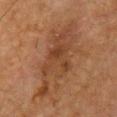The lesion was photographed on a routine skin check and not biopsied; there is no pathology result. This is a cross-polarized tile. Cropped from a total-body skin-imaging series; the visible field is about 15 mm. Automated image analysis of the tile measured a lesion area of about 33 mm², a shape eccentricity near 0.9, and a symmetry-axis asymmetry near 0.25. It also reported a mean CIELAB color near L≈34 a*≈17 b*≈27, a lesion–skin lightness drop of about 6, and a lesion-to-skin contrast of about 6 (normalized; higher = more distinct). The analysis additionally found a nevus-likeness score of about 0/100 and a lesion-detection confidence of about 90/100. The recorded lesion diameter is about 11 mm. A male patient, roughly 65 years of age. On the front of the torso.